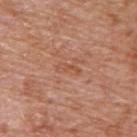Recorded during total-body skin imaging; not selected for excision or biopsy. This is a white-light tile. A 15 mm crop from a total-body photograph taken for skin-cancer surveillance. Approximately 2.5 mm at its widest. A male patient, roughly 65 years of age. Located on the upper back. The total-body-photography lesion software estimated an area of roughly 2.5 mm², an outline eccentricity of about 0.95 (0 = round, 1 = elongated), and two-axis asymmetry of about 0.45. The software also gave an automated nevus-likeness rating near 0 out of 100 and a lesion-detection confidence of about 95/100.